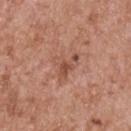tile lighting = white-light illumination | diameter = about 4 mm | imaging modality = total-body-photography crop, ~15 mm field of view | patient = male, aged around 60 | body site = the back | automated lesion analysis = an average lesion color of about L≈51 a*≈24 b*≈29 (CIELAB), about 9 CIELAB-L* units darker than the surrounding skin, and a normalized border contrast of about 6.5; a classifier nevus-likeness of about 0/100.